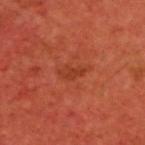The lesion was photographed on a routine skin check and not biopsied; there is no pathology result.
A 15 mm close-up extracted from a 3D total-body photography capture.
Approximately 3 mm at its widest.
The patient is a male aged 58 to 62.
On the upper back.
Captured under cross-polarized illumination.